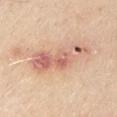{"site": "arm", "patient": {"sex": "male", "age_approx": 65}, "image": {"source": "total-body photography crop", "field_of_view_mm": 15}, "lighting": "white-light", "automated_metrics": {"cielab_L": 65, "cielab_a": 23, "cielab_b": 29, "vs_skin_darker_L": 12.0, "vs_skin_contrast_norm": 7.5, "nevus_likeness_0_100": 0, "lesion_detection_confidence_0_100": 100}, "lesion_size": {"long_diameter_mm_approx": 8.0}}